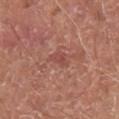Impression:
Recorded during total-body skin imaging; not selected for excision or biopsy.
Acquisition and patient details:
The lesion-visualizer software estimated an area of roughly 3.5 mm² and an eccentricity of roughly 0.9. The analysis additionally found an automated nevus-likeness rating near 0 out of 100. Imaged with white-light lighting. A region of skin cropped from a whole-body photographic capture, roughly 15 mm wide. About 3 mm across. Located on the right lower leg. A male patient, aged 63 to 67.